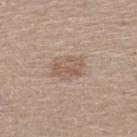biopsy status = catalogued during a skin exam; not biopsied
image = ~15 mm tile from a whole-body skin photo
subject = female, aged 68 to 72
lighting = white-light illumination
site = the left lower leg
size = about 3.5 mm
image-analysis metrics = a within-lesion color-variation index near 2.5/10